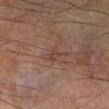Imaged during a routine full-body skin examination; the lesion was not biopsied and no histopathology is available. A region of skin cropped from a whole-body photographic capture, roughly 15 mm wide. A male patient, approximately 60 years of age. Longest diameter approximately 3.5 mm. Imaged with cross-polarized lighting. The lesion is located on the right lower leg. Automated tile analysis of the lesion measured a lesion color around L≈41 a*≈18 b*≈22 in CIELAB, a lesion–skin lightness drop of about 6, and a normalized border contrast of about 5.5. It also reported border irregularity of about 5.5 on a 0–10 scale and a within-lesion color-variation index near 2.5/10. The software also gave an automated nevus-likeness rating near 0 out of 100 and lesion-presence confidence of about 95/100.